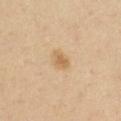Captured during whole-body skin photography for melanoma surveillance; the lesion was not biopsied.
A close-up tile cropped from a whole-body skin photograph, about 15 mm across.
Located on the right upper arm.
A male subject, about 55 years old.